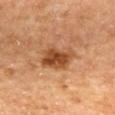follow-up: imaged on a skin check; not biopsied
image: total-body-photography crop, ~15 mm field of view
TBP lesion metrics: a border-irregularity index near 3/10, a within-lesion color-variation index near 5/10, and a peripheral color-asymmetry measure near 1.5
patient: female, aged 58–62
location: the upper back